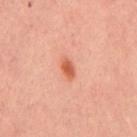  biopsy_status: not biopsied; imaged during a skin examination
  site: mid back
  lighting: cross-polarized
  image:
    source: total-body photography crop
    field_of_view_mm: 15
  lesion_size:
    long_diameter_mm_approx: 3.0
  patient:
    sex: male
    age_approx: 45